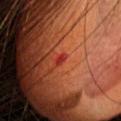Q: Patient demographics?
A: female, aged approximately 35
Q: What did automated image analysis measure?
A: a lesion area of about 1 mm² and a shape-asymmetry score of about 0.35 (0 = symmetric)
Q: How large is the lesion?
A: about 1.5 mm
Q: How was the tile lit?
A: cross-polarized illumination
Q: What kind of image is this?
A: ~15 mm tile from a whole-body skin photo
Q: What is the anatomic site?
A: the head or neck